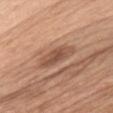Case summary:
- biopsy status · no biopsy performed (imaged during a skin exam)
- anatomic site · the chest
- automated metrics · a lesion area of about 7 mm² and a shape-asymmetry score of about 0.25 (0 = symmetric); an average lesion color of about L≈51 a*≈21 b*≈30 (CIELAB) and a lesion–skin lightness drop of about 10; a classifier nevus-likeness of about 25/100 and a lesion-detection confidence of about 95/100
- illumination · white-light illumination
- size · ≈5 mm
- patient · female, roughly 55 years of age
- image source · total-body-photography crop, ~15 mm field of view